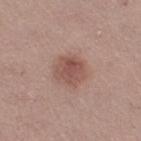- notes · total-body-photography surveillance lesion; no biopsy
- lesion diameter · ≈3.5 mm
- subject · female, aged 33–37
- body site · the leg
- imaging modality · ~15 mm tile from a whole-body skin photo
- lighting · white-light illumination